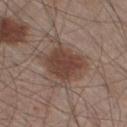diameter: ≈6.5 mm | subject: male, aged approximately 60 | site: the left lower leg | automated metrics: an average lesion color of about L≈43 a*≈17 b*≈24 (CIELAB), roughly 10 lightness units darker than nearby skin, and a normalized border contrast of about 8.5; border irregularity of about 5 on a 0–10 scale and a within-lesion color-variation index near 4/10 | image: total-body-photography crop, ~15 mm field of view.